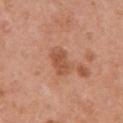{"biopsy_status": "not biopsied; imaged during a skin examination", "lesion_size": {"long_diameter_mm_approx": 3.5}, "image": {"source": "total-body photography crop", "field_of_view_mm": 15}, "lighting": "white-light", "automated_metrics": {"area_mm2_approx": 7.0, "shape_asymmetry": 0.25, "cielab_L": 53, "cielab_a": 25, "cielab_b": 33, "vs_skin_darker_L": 9.0, "vs_skin_contrast_norm": 6.5}, "site": "left upper arm", "patient": {"sex": "female", "age_approx": 40}}